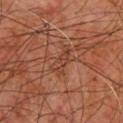- notes — total-body-photography surveillance lesion; no biopsy
- anatomic site — the upper back
- subject — male, aged 58 to 62
- tile lighting — cross-polarized
- lesion size — about 4 mm
- acquisition — 15 mm crop, total-body photography